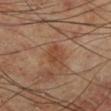The lesion-visualizer software estimated a border-irregularity rating of about 1.5/10, a within-lesion color-variation index near 3/10, and a peripheral color-asymmetry measure near 1. This is a cross-polarized tile. A male subject, approximately 70 years of age. The lesion is located on the left lower leg. Cropped from a whole-body photographic skin survey; the tile spans about 15 mm.